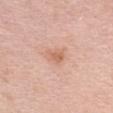{
  "biopsy_status": "not biopsied; imaged during a skin examination"
}